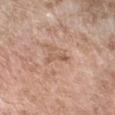No biopsy was performed on this lesion — it was imaged during a full skin examination and was not determined to be concerning. A female patient, aged 73–77. On the arm. The recorded lesion diameter is about 3 mm. Cropped from a whole-body photographic skin survey; the tile spans about 15 mm. This is a white-light tile.